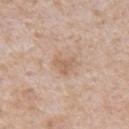Clinical impression:
No biopsy was performed on this lesion — it was imaged during a full skin examination and was not determined to be concerning.
Context:
The tile uses white-light illumination. The total-body-photography lesion software estimated a lesion area of about 4 mm² and a shape eccentricity near 0.55. And it measured a classifier nevus-likeness of about 0/100 and a detector confidence of about 100 out of 100 that the crop contains a lesion. This image is a 15 mm lesion crop taken from a total-body photograph. The lesion is on the mid back. A male subject aged 63 to 67. About 2.5 mm across.